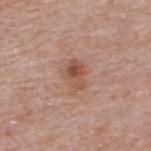On the upper back. This image is a 15 mm lesion crop taken from a total-body photograph. Longest diameter approximately 3.5 mm. The tile uses white-light illumination. An algorithmic analysis of the crop reported a mean CIELAB color near L≈53 a*≈21 b*≈28, a lesion–skin lightness drop of about 9, and a normalized lesion–skin contrast near 7. The software also gave a border-irregularity rating of about 3/10, a within-lesion color-variation index near 7.5/10, and a peripheral color-asymmetry measure near 3. And it measured a classifier nevus-likeness of about 55/100 and lesion-presence confidence of about 100/100. The patient is a male in their mid-50s.